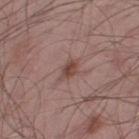workup = no biopsy performed (imaged during a skin exam)
size = ≈3 mm
subject = male, in their mid- to late 50s
image = total-body-photography crop, ~15 mm field of view
site = the right thigh
tile lighting = white-light illumination